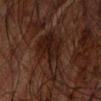The lesion was tiled from a total-body skin photograph and was not biopsied. An algorithmic analysis of the crop reported an area of roughly 15 mm², an outline eccentricity of about 0.75 (0 = round, 1 = elongated), and a shape-asymmetry score of about 0.5 (0 = symmetric). It also reported an average lesion color of about L≈15 a*≈15 b*≈17 (CIELAB), a lesion–skin lightness drop of about 6, and a lesion-to-skin contrast of about 9 (normalized; higher = more distinct). The software also gave a border-irregularity rating of about 5/10, internal color variation of about 4.5 on a 0–10 scale, and radial color variation of about 1.5. A male patient about 65 years old. The lesion is on the arm. A 15 mm close-up extracted from a 3D total-body photography capture. Longest diameter approximately 6 mm.